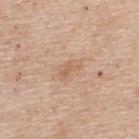Clinical impression: The lesion was tiled from a total-body skin photograph and was not biopsied. Context: Cropped from a total-body skin-imaging series; the visible field is about 15 mm. The subject is a male approximately 55 years of age. From the upper back.